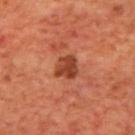No biopsy was performed on this lesion — it was imaged during a full skin examination and was not determined to be concerning. A region of skin cropped from a whole-body photographic capture, roughly 15 mm wide. This is a cross-polarized tile. Measured at roughly 3 mm in maximum diameter. The subject is a male roughly 70 years of age. The lesion-visualizer software estimated an area of roughly 6.5 mm², an outline eccentricity of about 0.3 (0 = round, 1 = elongated), and a shape-asymmetry score of about 0.25 (0 = symmetric). And it measured a lesion color around L≈42 a*≈30 b*≈34 in CIELAB. The software also gave internal color variation of about 3.5 on a 0–10 scale and radial color variation of about 1.5. The lesion is located on the upper back.